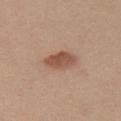<lesion>
<site>chest</site>
<lighting>white-light</lighting>
<patient>
  <sex>female</sex>
  <age_approx>20</age_approx>
</patient>
<image>
  <source>total-body photography crop</source>
  <field_of_view_mm>15</field_of_view_mm>
</image>
</lesion>